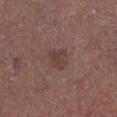Findings:
– workup · total-body-photography surveillance lesion; no biopsy
– location · the leg
– subject · male, roughly 65 years of age
– size · ≈3 mm
– tile lighting · white-light
– TBP lesion metrics · a lesion area of about 4.5 mm² and a shape eccentricity near 0.55; an average lesion color of about L≈39 a*≈18 b*≈20 (CIELAB) and roughly 7 lightness units darker than nearby skin; a border-irregularity rating of about 3.5/10 and radial color variation of about 0.5
– image source · 15 mm crop, total-body photography